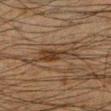workup = total-body-photography surveillance lesion; no biopsy
subject = male, roughly 35 years of age
image = total-body-photography crop, ~15 mm field of view
site = the left lower leg
lighting = cross-polarized
diameter = ≈6 mm